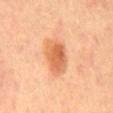Recorded during total-body skin imaging; not selected for excision or biopsy. A male subject, approximately 70 years of age. Approximately 4.5 mm at its widest. A close-up tile cropped from a whole-body skin photograph, about 15 mm across. From the mid back.